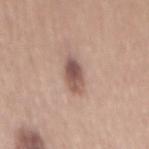notes: imaged on a skin check; not biopsied
subject: male, aged 53 to 57
site: the mid back
image: ~15 mm tile from a whole-body skin photo